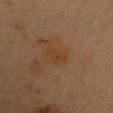Assessment: Captured during whole-body skin photography for melanoma surveillance; the lesion was not biopsied. Background: The lesion is located on the chest. A female patient, aged 38 to 42. A 15 mm close-up tile from a total-body photography series done for melanoma screening.